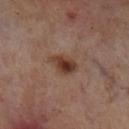A 15 mm close-up extracted from a 3D total-body photography capture.
On the left lower leg.
A male patient, aged around 70.
The recorded lesion diameter is about 3.5 mm.